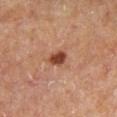The lesion was tiled from a total-body skin photograph and was not biopsied. An algorithmic analysis of the crop reported an average lesion color of about L≈39 a*≈24 b*≈28 (CIELAB), roughly 14 lightness units darker than nearby skin, and a lesion-to-skin contrast of about 11 (normalized; higher = more distinct). The software also gave border irregularity of about 2 on a 0–10 scale, a color-variation rating of about 2/10, and a peripheral color-asymmetry measure near 0.5. It also reported an automated nevus-likeness rating near 95 out of 100 and a detector confidence of about 100 out of 100 that the crop contains a lesion. The lesion is on the left lower leg. A 15 mm crop from a total-body photograph taken for skin-cancer surveillance. A male patient, aged 63–67. Imaged with cross-polarized lighting.